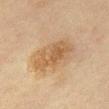| feature | finding |
|---|---|
| biopsy status | imaged on a skin check; not biopsied |
| imaging modality | ~15 mm tile from a whole-body skin photo |
| size | about 6 mm |
| subject | female, roughly 65 years of age |
| anatomic site | the front of the torso |
| tile lighting | cross-polarized |